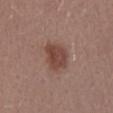Findings:
* follow-up: imaged on a skin check; not biopsied
* location: the lower back
* size: ≈3.5 mm
* subject: female, roughly 25 years of age
* imaging modality: 15 mm crop, total-body photography
* illumination: white-light illumination